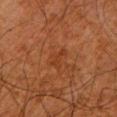Impression: The lesion was photographed on a routine skin check and not biopsied; there is no pathology result. Acquisition and patient details: An algorithmic analysis of the crop reported a footprint of about 3 mm², an eccentricity of roughly 0.9, and a shape-asymmetry score of about 0.4 (0 = symmetric). And it measured a lesion–skin lightness drop of about 4. It also reported a border-irregularity rating of about 4.5/10, a within-lesion color-variation index near 0/10, and peripheral color asymmetry of about 0. On the leg. The patient is a male aged 78–82. Measured at roughly 3 mm in maximum diameter. A 15 mm close-up extracted from a 3D total-body photography capture.